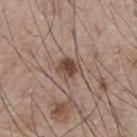Q: Was this lesion biopsied?
A: imaged on a skin check; not biopsied
Q: Patient demographics?
A: male, aged 73 to 77
Q: How was this image acquired?
A: ~15 mm tile from a whole-body skin photo
Q: How large is the lesion?
A: ~3 mm (longest diameter)
Q: Where on the body is the lesion?
A: the chest
Q: How was the tile lit?
A: white-light illumination
Q: What did automated image analysis measure?
A: a footprint of about 5.5 mm², an outline eccentricity of about 0.55 (0 = round, 1 = elongated), and two-axis asymmetry of about 0.2; internal color variation of about 4.5 on a 0–10 scale and a peripheral color-asymmetry measure near 1.5; a nevus-likeness score of about 95/100 and a lesion-detection confidence of about 100/100